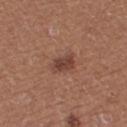Impression:
Recorded during total-body skin imaging; not selected for excision or biopsy.
Image and clinical context:
The lesion is located on the right thigh. Cropped from a whole-body photographic skin survey; the tile spans about 15 mm. A female subject, aged approximately 35. The tile uses white-light illumination. The lesion-visualizer software estimated an area of roughly 4.5 mm², a shape eccentricity near 0.85, and a shape-asymmetry score of about 0.25 (0 = symmetric). It also reported a border-irregularity rating of about 2.5/10 and peripheral color asymmetry of about 1.